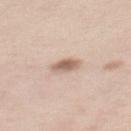From the upper back.
A female patient approximately 35 years of age.
A close-up tile cropped from a whole-body skin photograph, about 15 mm across.
Longest diameter approximately 3 mm.